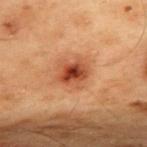biopsy status = total-body-photography surveillance lesion; no biopsy